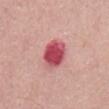workup: imaged on a skin check; not biopsied | acquisition: 15 mm crop, total-body photography | illumination: white-light illumination | size: ~3.5 mm (longest diameter) | subject: male, approximately 55 years of age.